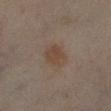| key | value |
|---|---|
| workup | catalogued during a skin exam; not biopsied |
| image source | 15 mm crop, total-body photography |
| subject | female, aged approximately 60 |
| location | the right lower leg |
| tile lighting | cross-polarized illumination |
| image-analysis metrics | an area of roughly 8 mm², an eccentricity of roughly 0.7, and a shape-asymmetry score of about 0.2 (0 = symmetric); a border-irregularity index near 1.5/10, a color-variation rating of about 2.5/10, and a peripheral color-asymmetry measure near 1; a nevus-likeness score of about 35/100 and lesion-presence confidence of about 100/100 |